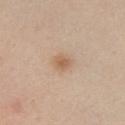No biopsy was performed on this lesion — it was imaged during a full skin examination and was not determined to be concerning. A female subject roughly 45 years of age. The lesion is on the front of the torso. An algorithmic analysis of the crop reported a lesion color around L≈60 a*≈19 b*≈32 in CIELAB, about 9 CIELAB-L* units darker than the surrounding skin, and a normalized lesion–skin contrast near 6.5. And it measured internal color variation of about 2 on a 0–10 scale and peripheral color asymmetry of about 1. A close-up tile cropped from a whole-body skin photograph, about 15 mm across. Approximately 2 mm at its widest. The tile uses white-light illumination.